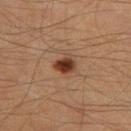Q: Was this lesion biopsied?
A: no biopsy performed (imaged during a skin exam)
Q: Illumination type?
A: cross-polarized illumination
Q: Automated lesion metrics?
A: a border-irregularity rating of about 2/10 and peripheral color asymmetry of about 1
Q: What kind of image is this?
A: total-body-photography crop, ~15 mm field of view
Q: Who is the patient?
A: male, approximately 50 years of age
Q: Lesion location?
A: the leg